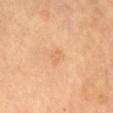Imaged during a routine full-body skin examination; the lesion was not biopsied and no histopathology is available.